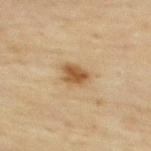Q: Is there a histopathology result?
A: catalogued during a skin exam; not biopsied
Q: Automated lesion metrics?
A: a shape eccentricity near 0.5 and a shape-asymmetry score of about 0.25 (0 = symmetric); a mean CIELAB color near L≈47 a*≈16 b*≈33, a lesion–skin lightness drop of about 11, and a lesion-to-skin contrast of about 8.5 (normalized; higher = more distinct)
Q: What is the anatomic site?
A: the upper back
Q: How was the tile lit?
A: cross-polarized
Q: What kind of image is this?
A: total-body-photography crop, ~15 mm field of view
Q: What are the patient's age and sex?
A: male, aged around 45